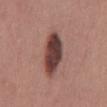Recorded during total-body skin imaging; not selected for excision or biopsy. This image is a 15 mm lesion crop taken from a total-body photograph. From the mid back. The tile uses white-light illumination. Longest diameter approximately 6.5 mm. Automated tile analysis of the lesion measured an outline eccentricity of about 0.9 (0 = round, 1 = elongated) and a symmetry-axis asymmetry near 0.15. A male subject roughly 40 years of age.